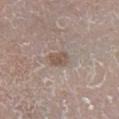follow-up = no biopsy performed (imaged during a skin exam)
tile lighting = white-light illumination
body site = the leg
patient = male, aged 58 to 62
lesion diameter = about 2.5 mm
automated metrics = an area of roughly 3.5 mm², a shape eccentricity near 0.75, and two-axis asymmetry of about 0.2; an automated nevus-likeness rating near 40 out of 100 and lesion-presence confidence of about 95/100
image = ~15 mm tile from a whole-body skin photo